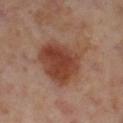Part of a total-body skin-imaging series; this lesion was reviewed on a skin check and was not flagged for biopsy. On the right lower leg. Measured at roughly 6.5 mm in maximum diameter. Captured under cross-polarized illumination. Cropped from a whole-body photographic skin survey; the tile spans about 15 mm. The patient is a female approximately 55 years of age.